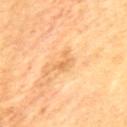A roughly 15 mm field-of-view crop from a total-body skin photograph. About 3 mm across. The lesion is located on the mid back. A male subject, about 60 years old.